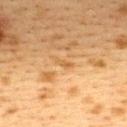This lesion was catalogued during total-body skin photography and was not selected for biopsy. The subject is a female about 40 years old. Captured under cross-polarized illumination. The lesion-visualizer software estimated a color-variation rating of about 0/10. And it measured an automated nevus-likeness rating near 0 out of 100 and a detector confidence of about 85 out of 100 that the crop contains a lesion. Longest diameter approximately 2.5 mm. Cropped from a whole-body photographic skin survey; the tile spans about 15 mm. The lesion is on the upper back.